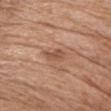Located on the chest.
Approximately 2.5 mm at its widest.
Captured under white-light illumination.
Cropped from a whole-body photographic skin survey; the tile spans about 15 mm.
Automated image analysis of the tile measured a lesion area of about 3.5 mm², a shape eccentricity near 0.75, and two-axis asymmetry of about 0.3. The software also gave a mean CIELAB color near L≈52 a*≈21 b*≈31 and a lesion-to-skin contrast of about 6 (normalized; higher = more distinct).
The subject is a male in their 70s.